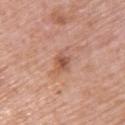notes: no biopsy performed (imaged during a skin exam) | lighting: white-light illumination | subject: female, approximately 60 years of age | body site: the upper back | automated lesion analysis: roughly 10 lightness units darker than nearby skin and a normalized lesion–skin contrast near 6.5; an automated nevus-likeness rating near 15 out of 100 and a lesion-detection confidence of about 100/100 | acquisition: ~15 mm tile from a whole-body skin photo | size: about 3 mm.